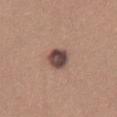follow-up: total-body-photography surveillance lesion; no biopsy
lesion diameter: about 3.5 mm
image: ~15 mm crop, total-body skin-cancer survey
illumination: white-light
location: the left thigh
patient: female, aged 33–37